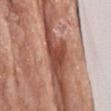Recorded during total-body skin imaging; not selected for excision or biopsy. The tile uses white-light illumination. On the left forearm. The subject is a female aged around 75. A lesion tile, about 15 mm wide, cut from a 3D total-body photograph. About 4.5 mm across.